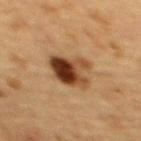Part of a total-body skin-imaging series; this lesion was reviewed on a skin check and was not flagged for biopsy. The total-body-photography lesion software estimated a footprint of about 13 mm², a shape eccentricity near 0.6, and a shape-asymmetry score of about 0.2 (0 = symmetric). And it measured a border-irregularity index near 2.5/10 and a peripheral color-asymmetry measure near 5. The recorded lesion diameter is about 5 mm. Captured under cross-polarized illumination. The patient is a female aged 68 to 72. On the upper back. Cropped from a total-body skin-imaging series; the visible field is about 15 mm.